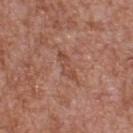  biopsy_status: not biopsied; imaged during a skin examination
  patient:
    sex: male
    age_approx: 65
  lesion_size:
    long_diameter_mm_approx: 3.5
  site: upper back
  image:
    source: total-body photography crop
    field_of_view_mm: 15
  lighting: white-light